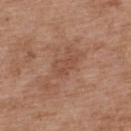biopsy status: catalogued during a skin exam; not biopsied
site: the upper back
patient: female, aged around 40
image source: ~15 mm crop, total-body skin-cancer survey
lighting: white-light illumination